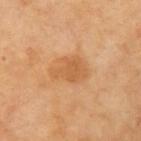{"site": "left upper arm", "patient": {"sex": "male", "age_approx": 70}, "lesion_size": {"long_diameter_mm_approx": 4.5}, "image": {"source": "total-body photography crop", "field_of_view_mm": 15}, "lighting": "cross-polarized"}